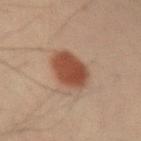This lesion was catalogued during total-body skin photography and was not selected for biopsy. Located on the left forearm. Captured under cross-polarized illumination. A male patient approximately 40 years of age. A 15 mm close-up extracted from a 3D total-body photography capture.